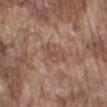biopsy status — total-body-photography surveillance lesion; no biopsy.